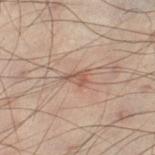| key | value |
|---|---|
| follow-up | no biopsy performed (imaged during a skin exam) |
| automated metrics | a lesion area of about 3 mm², an outline eccentricity of about 0.8 (0 = round, 1 = elongated), and a shape-asymmetry score of about 0.4 (0 = symmetric); a border-irregularity rating of about 3.5/10, a within-lesion color-variation index near 1.5/10, and radial color variation of about 0.5; an automated nevus-likeness rating near 0 out of 100 and a lesion-detection confidence of about 100/100 |
| acquisition | total-body-photography crop, ~15 mm field of view |
| anatomic site | the leg |
| patient | male, aged 48–52 |
| size | ≈2.5 mm |